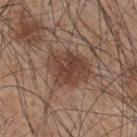  biopsy_status: not biopsied; imaged during a skin examination
  site: upper back
  image:
    source: total-body photography crop
    field_of_view_mm: 15
  patient:
    sex: male
    age_approx: 45
  automated_metrics:
    cielab_L: 42
    cielab_a: 19
    cielab_b: 26
    vs_skin_darker_L: 10.0
    border_irregularity_0_10: 3.0
    color_variation_0_10: 4.0
    peripheral_color_asymmetry: 1.5
    nevus_likeness_0_100: 35
    lesion_detection_confidence_0_100: 100
  lighting: white-light